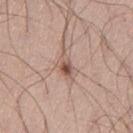The total-body-photography lesion software estimated an area of roughly 6 mm² and two-axis asymmetry of about 0.45. It also reported a border-irregularity index near 5.5/10, a within-lesion color-variation index near 9/10, and peripheral color asymmetry of about 3. The software also gave an automated nevus-likeness rating near 25 out of 100 and a lesion-detection confidence of about 100/100.
A 15 mm close-up tile from a total-body photography series done for melanoma screening.
Located on the left thigh.
Longest diameter approximately 4.5 mm.
A male subject aged approximately 30.